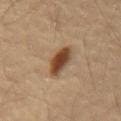biopsy_status: not biopsied; imaged during a skin examination
patient:
  sex: male
  age_approx: 55
image:
  source: total-body photography crop
  field_of_view_mm: 15
lighting: cross-polarized
site: abdomen